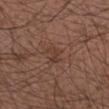Q: Is there a histopathology result?
A: no biopsy performed (imaged during a skin exam)
Q: Automated lesion metrics?
A: an area of roughly 4 mm², a shape eccentricity near 0.65, and two-axis asymmetry of about 0.3
Q: What is the lesion's diameter?
A: ~2.5 mm (longest diameter)
Q: What is the anatomic site?
A: the right forearm
Q: What is the imaging modality?
A: 15 mm crop, total-body photography
Q: Patient demographics?
A: male, roughly 55 years of age
Q: What lighting was used for the tile?
A: white-light